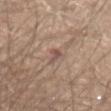This lesion was catalogued during total-body skin photography and was not selected for biopsy. Imaged with white-light lighting. Cropped from a whole-body photographic skin survey; the tile spans about 15 mm. The subject is a male approximately 65 years of age. The recorded lesion diameter is about 3 mm. On the abdomen.